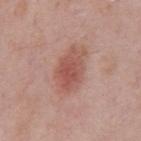Q: Is there a histopathology result?
A: total-body-photography surveillance lesion; no biopsy
Q: What is the lesion's diameter?
A: ≈5.5 mm
Q: What is the imaging modality?
A: total-body-photography crop, ~15 mm field of view
Q: What is the anatomic site?
A: the front of the torso
Q: Patient demographics?
A: male, about 70 years old